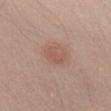The lesion was tiled from a total-body skin photograph and was not biopsied. A 15 mm close-up extracted from a 3D total-body photography capture. The lesion is on the abdomen. An algorithmic analysis of the crop reported border irregularity of about 3 on a 0–10 scale, a within-lesion color-variation index near 1.5/10, and a peripheral color-asymmetry measure near 0.5. Captured under white-light illumination. The subject is a male aged 38–42. The recorded lesion diameter is about 2.5 mm.